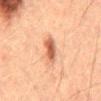Assessment: Imaged during a routine full-body skin examination; the lesion was not biopsied and no histopathology is available. Context: An algorithmic analysis of the crop reported roughly 12 lightness units darker than nearby skin and a normalized lesion–skin contrast near 9. And it measured a classifier nevus-likeness of about 95/100. Longest diameter approximately 4 mm. A male patient, aged approximately 50. Captured under cross-polarized illumination. On the mid back. A 15 mm close-up tile from a total-body photography series done for melanoma screening.